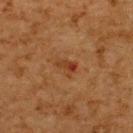Case summary:
• biopsy status: total-body-photography surveillance lesion; no biopsy
• diameter: ~2.5 mm (longest diameter)
• patient: male, aged around 60
• image: 15 mm crop, total-body photography
• lighting: cross-polarized illumination
• site: the upper back